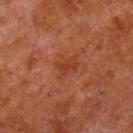The lesion was tiled from a total-body skin photograph and was not biopsied.
A lesion tile, about 15 mm wide, cut from a 3D total-body photograph.
A male subject aged 78 to 82.
Approximately 3 mm at its widest.
On the left lower leg.
The tile uses cross-polarized illumination.